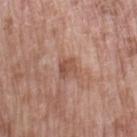Impression:
Recorded during total-body skin imaging; not selected for excision or biopsy.
Acquisition and patient details:
From the left upper arm. This image is a 15 mm lesion crop taken from a total-body photograph. A female patient, approximately 70 years of age. The tile uses white-light illumination.